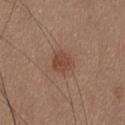Clinical impression:
Imaged during a routine full-body skin examination; the lesion was not biopsied and no histopathology is available.
Context:
The tile uses white-light illumination. The lesion is located on the head or neck. A female subject, aged around 35. Cropped from a whole-body photographic skin survey; the tile spans about 15 mm. The lesion's longest dimension is about 2.5 mm.